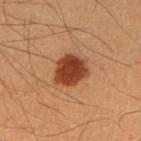notes = no biopsy performed (imaged during a skin exam)
anatomic site = the arm
acquisition = ~15 mm tile from a whole-body skin photo
patient = male, aged around 35
diameter = ≈4 mm
tile lighting = cross-polarized illumination